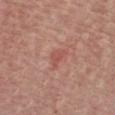Imaged during a routine full-body skin examination; the lesion was not biopsied and no histopathology is available.
A lesion tile, about 15 mm wide, cut from a 3D total-body photograph.
Automated tile analysis of the lesion measured a border-irregularity index near 4/10 and radial color variation of about 0.5. The analysis additionally found a nevus-likeness score of about 0/100 and lesion-presence confidence of about 100/100.
Imaged with white-light lighting.
On the front of the torso.
Approximately 2.5 mm at its widest.
A male patient, aged around 65.